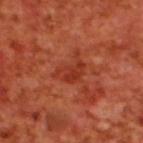Recorded during total-body skin imaging; not selected for excision or biopsy.
The tile uses cross-polarized illumination.
A male patient in their 70s.
Cropped from a whole-body photographic skin survey; the tile spans about 15 mm.
The lesion's longest dimension is about 4 mm.
From the back.